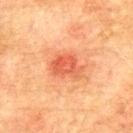A male subject, in their mid- to late 70s.
Imaged with cross-polarized lighting.
A roughly 15 mm field-of-view crop from a total-body skin photograph.
The lesion-visualizer software estimated a mean CIELAB color near L≈51 a*≈31 b*≈35 and roughly 10 lightness units darker than nearby skin. The software also gave a border-irregularity index near 3/10, internal color variation of about 5.5 on a 0–10 scale, and peripheral color asymmetry of about 2.
Longest diameter approximately 4 mm.
Located on the upper back.